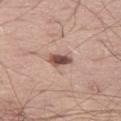Clinical impression:
No biopsy was performed on this lesion — it was imaged during a full skin examination and was not determined to be concerning.
Image and clinical context:
A 15 mm close-up extracted from a 3D total-body photography capture. The subject is a male aged around 60. Longest diameter approximately 3 mm. This is a white-light tile. Located on the left thigh.